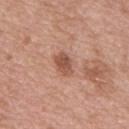Clinical impression:
No biopsy was performed on this lesion — it was imaged during a full skin examination and was not determined to be concerning.
Context:
This is a white-light tile. A male subject, about 50 years old. Cropped from a whole-body photographic skin survey; the tile spans about 15 mm. Measured at roughly 3 mm in maximum diameter. Located on the mid back.